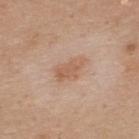Case summary:
• workup · catalogued during a skin exam; not biopsied
• site · the upper back
• image · 15 mm crop, total-body photography
• automated metrics · an area of roughly 7 mm², an eccentricity of roughly 0.8, and two-axis asymmetry of about 0.3; a lesion color around L≈59 a*≈20 b*≈31 in CIELAB, roughly 8 lightness units darker than nearby skin, and a normalized lesion–skin contrast near 6; a nevus-likeness score of about 40/100 and lesion-presence confidence of about 100/100
• subject · male, aged around 35